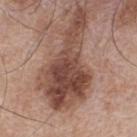The lesion was tiled from a total-body skin photograph and was not biopsied.
From the upper back.
Approximately 11.5 mm at its widest.
A 15 mm close-up extracted from a 3D total-body photography capture.
A male patient in their mid-60s.